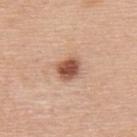The lesion was tiled from a total-body skin photograph and was not biopsied. The lesion is on the upper back. This is a white-light tile. A male subject aged approximately 50. The recorded lesion diameter is about 3 mm. A lesion tile, about 15 mm wide, cut from a 3D total-body photograph.